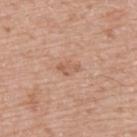Notes:
* notes: imaged on a skin check; not biopsied
* body site: the upper back
* TBP lesion metrics: an area of roughly 4 mm² and an outline eccentricity of about 0.8 (0 = round, 1 = elongated); an average lesion color of about L≈59 a*≈21 b*≈30 (CIELAB) and roughly 7 lightness units darker than nearby skin; an automated nevus-likeness rating near 0 out of 100 and lesion-presence confidence of about 100/100
* patient: male, aged 73 to 77
* tile lighting: white-light
* lesion diameter: ~3 mm (longest diameter)
* image source: ~15 mm crop, total-body skin-cancer survey